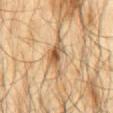The lesion was photographed on a routine skin check and not biopsied; there is no pathology result. A lesion tile, about 15 mm wide, cut from a 3D total-body photograph. The lesion is on the mid back. The subject is a male roughly 65 years of age.